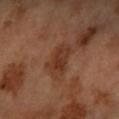Q: Was a biopsy performed?
A: no biopsy performed (imaged during a skin exam)
Q: Where on the body is the lesion?
A: the left forearm
Q: What lighting was used for the tile?
A: cross-polarized
Q: How was this image acquired?
A: 15 mm crop, total-body photography
Q: What are the patient's age and sex?
A: female, aged 58–62
Q: How large is the lesion?
A: ~4 mm (longest diameter)